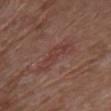Impression:
Captured during whole-body skin photography for melanoma surveillance; the lesion was not biopsied.
Context:
The patient is a female in their 80s. The lesion is located on the chest. Cropped from a whole-body photographic skin survey; the tile spans about 15 mm.